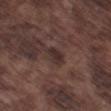Captured during whole-body skin photography for melanoma surveillance; the lesion was not biopsied.
Longest diameter approximately 2.5 mm.
A region of skin cropped from a whole-body photographic capture, roughly 15 mm wide.
The lesion-visualizer software estimated an average lesion color of about L≈29 a*≈15 b*≈17 (CIELAB) and a lesion-to-skin contrast of about 8 (normalized; higher = more distinct). It also reported a border-irregularity rating of about 2/10, a within-lesion color-variation index near 1.5/10, and peripheral color asymmetry of about 0.5.
Imaged with white-light lighting.
On the left thigh.
The subject is a male aged around 75.